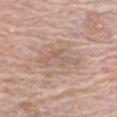workup: no biopsy performed (imaged during a skin exam); body site: the chest; illumination: white-light; subject: male, roughly 80 years of age; acquisition: ~15 mm crop, total-body skin-cancer survey.